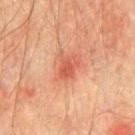Q: Was this lesion biopsied?
A: catalogued during a skin exam; not biopsied
Q: Illumination type?
A: cross-polarized illumination
Q: What is the anatomic site?
A: the front of the torso
Q: Who is the patient?
A: male, aged approximately 75
Q: How large is the lesion?
A: ≈3 mm
Q: How was this image acquired?
A: 15 mm crop, total-body photography
Q: Automated lesion metrics?
A: a shape-asymmetry score of about 0.3 (0 = symmetric)